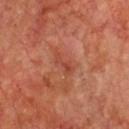  patient:
    sex: male
    age_approx: 70
  lesion_size:
    long_diameter_mm_approx: 2.5
  site: chest
  image:
    source: total-body photography crop
    field_of_view_mm: 15
  automated_metrics:
    area_mm2_approx: 2.5
    eccentricity: 0.95
    cielab_L: 44
    cielab_a: 30
    cielab_b: 32
    vs_skin_darker_L: 7.0
    lesion_detection_confidence_0_100: 80